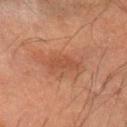notes = catalogued during a skin exam; not biopsied
site = the arm
imaging modality = total-body-photography crop, ~15 mm field of view
patient = male, in their mid- to late 60s
illumination = cross-polarized illumination
automated metrics = a lesion area of about 4 mm² and a symmetry-axis asymmetry near 0.35; a nevus-likeness score of about 0/100